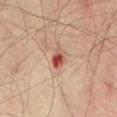Notes:
- workup — total-body-photography surveillance lesion; no biopsy
- subject — male, about 45 years old
- automated metrics — an outline eccentricity of about 0.8 (0 = round, 1 = elongated); roughly 13 lightness units darker than nearby skin and a normalized border contrast of about 9.5; border irregularity of about 2.5 on a 0–10 scale and radial color variation of about 2.5; a nevus-likeness score of about 0/100 and a lesion-detection confidence of about 100/100
- lighting — cross-polarized
- image — 15 mm crop, total-body photography
- body site — the abdomen